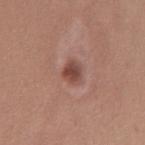The lesion was photographed on a routine skin check and not biopsied; there is no pathology result. Cropped from a total-body skin-imaging series; the visible field is about 15 mm. The subject is a female aged 33–37. Captured under white-light illumination. From the mid back.